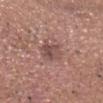Assessment:
The lesion was tiled from a total-body skin photograph and was not biopsied.
Context:
A male patient, in their mid- to late 70s. A close-up tile cropped from a whole-body skin photograph, about 15 mm across. From the head or neck.